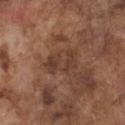Imaged during a routine full-body skin examination; the lesion was not biopsied and no histopathology is available. This image is a 15 mm lesion crop taken from a total-body photograph. Imaged with white-light lighting. A male subject aged around 75. The lesion is located on the chest.